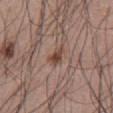This lesion was catalogued during total-body skin photography and was not selected for biopsy. A male patient in their mid- to late 50s. The lesion is on the abdomen. The total-body-photography lesion software estimated an area of roughly 4 mm², an eccentricity of roughly 0.8, and a shape-asymmetry score of about 0.25 (0 = symmetric). The analysis additionally found a lesion color around L≈46 a*≈19 b*≈26 in CIELAB, about 10 CIELAB-L* units darker than the surrounding skin, and a normalized lesion–skin contrast near 8. The software also gave an automated nevus-likeness rating near 90 out of 100 and a lesion-detection confidence of about 100/100. Cropped from a total-body skin-imaging series; the visible field is about 15 mm.